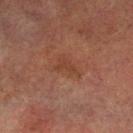follow-up: catalogued during a skin exam; not biopsied | patient: male, in their mid-70s | size: about 3 mm | site: the left lower leg | imaging modality: total-body-photography crop, ~15 mm field of view.